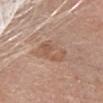Part of a total-body skin-imaging series; this lesion was reviewed on a skin check and was not flagged for biopsy. This is a white-light tile. A female patient, roughly 70 years of age. A close-up tile cropped from a whole-body skin photograph, about 15 mm across. Longest diameter approximately 4 mm. The lesion-visualizer software estimated an area of roughly 10 mm² and two-axis asymmetry of about 0.25. The analysis additionally found a mean CIELAB color near L≈56 a*≈19 b*≈29, a lesion–skin lightness drop of about 8, and a lesion-to-skin contrast of about 5.5 (normalized; higher = more distinct). It also reported an automated nevus-likeness rating near 0 out of 100 and lesion-presence confidence of about 100/100. The lesion is located on the head or neck.